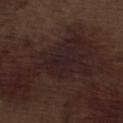{"image": {"source": "total-body photography crop", "field_of_view_mm": 15}, "lighting": "white-light", "lesion_size": {"long_diameter_mm_approx": 4.0}, "automated_metrics": {"area_mm2_approx": 7.5, "eccentricity": 0.8, "shape_asymmetry": 0.35, "cielab_L": 19, "cielab_a": 14, "cielab_b": 12, "vs_skin_darker_L": 4.0, "vs_skin_contrast_norm": 7.5, "nevus_likeness_0_100": 0, "lesion_detection_confidence_0_100": 55}, "patient": {"sex": "male", "age_approx": 70}, "site": "left lower leg"}